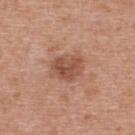Case summary:
• acquisition — ~15 mm crop, total-body skin-cancer survey
• site — the upper back
• patient — female, aged around 40
• lighting — white-light illumination
• lesion size — ~4 mm (longest diameter)